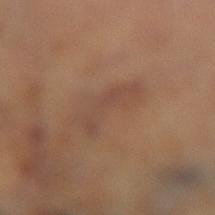Case summary:
* follow-up: imaged on a skin check; not biopsied
* illumination: cross-polarized illumination
* image: ~15 mm crop, total-body skin-cancer survey
* location: the left lower leg